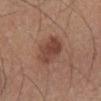Q: Is there a histopathology result?
A: imaged on a skin check; not biopsied
Q: What did automated image analysis measure?
A: an area of roughly 11 mm², an eccentricity of roughly 0.55, and a shape-asymmetry score of about 0.2 (0 = symmetric); an automated nevus-likeness rating near 75 out of 100 and a lesion-detection confidence of about 100/100
Q: How large is the lesion?
A: ~4 mm (longest diameter)
Q: Patient demographics?
A: male, aged 43–47
Q: Lesion location?
A: the right lower leg
Q: What is the imaging modality?
A: 15 mm crop, total-body photography
Q: What lighting was used for the tile?
A: white-light illumination